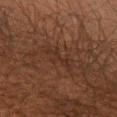{
  "biopsy_status": "not biopsied; imaged during a skin examination",
  "site": "arm",
  "patient": {
    "sex": "male",
    "age_approx": 50
  },
  "image": {
    "source": "total-body photography crop",
    "field_of_view_mm": 15
  },
  "lesion_size": {
    "long_diameter_mm_approx": 3.5
  }
}